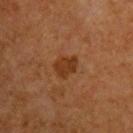| key | value |
|---|---|
| subject | male, aged around 65 |
| image source | 15 mm crop, total-body photography |
| site | the chest |
| diameter | ~3 mm (longest diameter) |
| TBP lesion metrics | a footprint of about 5.5 mm²; a lesion color around L≈30 a*≈21 b*≈31 in CIELAB, a lesion–skin lightness drop of about 8, and a lesion-to-skin contrast of about 8 (normalized; higher = more distinct); a peripheral color-asymmetry measure near 0.5; a classifier nevus-likeness of about 55/100 and lesion-presence confidence of about 100/100 |
| illumination | cross-polarized illumination |